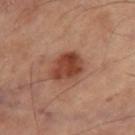Impression:
The lesion was photographed on a routine skin check and not biopsied; there is no pathology result.
Acquisition and patient details:
From the right thigh. A 15 mm crop from a total-body photograph taken for skin-cancer surveillance. Longest diameter approximately 4.5 mm.